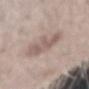lighting: white-light
image:
  source: total-body photography crop
  field_of_view_mm: 15
site: right forearm
patient:
  sex: male
  age_approx: 30
lesion_size:
  long_diameter_mm_approx: 4.0